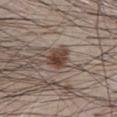workup: total-body-photography surveillance lesion; no biopsy
patient: male, in their 80s
acquisition: ~15 mm crop, total-body skin-cancer survey
body site: the abdomen